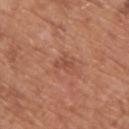Assessment: Part of a total-body skin-imaging series; this lesion was reviewed on a skin check and was not flagged for biopsy.